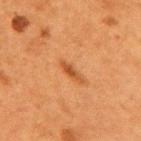Captured during whole-body skin photography for melanoma surveillance; the lesion was not biopsied.
Located on the mid back.
A female patient aged 48 to 52.
Measured at roughly 2.5 mm in maximum diameter.
The tile uses cross-polarized illumination.
A 15 mm close-up extracted from a 3D total-body photography capture.